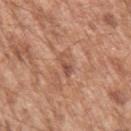| key | value |
|---|---|
| notes | no biopsy performed (imaged during a skin exam) |
| image source | ~15 mm tile from a whole-body skin photo |
| patient | male, in their mid-60s |
| location | the left upper arm |